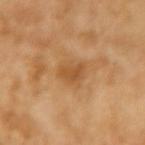This lesion was catalogued during total-body skin photography and was not selected for biopsy.
Located on the left forearm.
A female patient, in their 70s.
This image is a 15 mm lesion crop taken from a total-body photograph.
The tile uses cross-polarized illumination.
The recorded lesion diameter is about 3.5 mm.